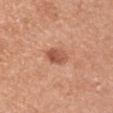Recorded during total-body skin imaging; not selected for excision or biopsy.
A region of skin cropped from a whole-body photographic capture, roughly 15 mm wide.
This is a white-light tile.
Located on the left upper arm.
The lesion's longest dimension is about 3.5 mm.
The subject is a female aged 48–52.